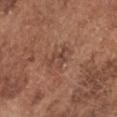| field | value |
|---|---|
| workup | total-body-photography surveillance lesion; no biopsy |
| tile lighting | white-light |
| subject | male, aged around 75 |
| body site | the head or neck |
| image | total-body-photography crop, ~15 mm field of view |